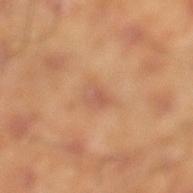Context:
A male subject, approximately 45 years of age. The recorded lesion diameter is about 3 mm. This image is a 15 mm lesion crop taken from a total-body photograph. From the leg. The tile uses cross-polarized illumination.